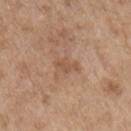  lighting: white-light
  image:
    source: total-body photography crop
    field_of_view_mm: 15
  automated_metrics:
    border_irregularity_0_10: 4.0
    color_variation_0_10: 0.5
    peripheral_color_asymmetry: 0.0
    nevus_likeness_0_100: 0
    lesion_detection_confidence_0_100: 100
  patient:
    sex: female
    age_approx: 85+
  site: left upper arm
  lesion_size:
    long_diameter_mm_approx: 2.5